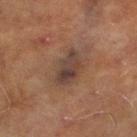Imaged during a routine full-body skin examination; the lesion was not biopsied and no histopathology is available. This is a cross-polarized tile. The recorded lesion diameter is about 4 mm. Cropped from a total-body skin-imaging series; the visible field is about 15 mm. On the right lower leg. The patient is a male aged around 70. The lesion-visualizer software estimated an area of roughly 8.5 mm² and an eccentricity of roughly 0.8. It also reported an average lesion color of about L≈32 a*≈14 b*≈20 (CIELAB), roughly 8 lightness units darker than nearby skin, and a normalized lesion–skin contrast near 8.5. The software also gave a nevus-likeness score of about 0/100 and a lesion-detection confidence of about 100/100.